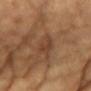follow-up: no biopsy performed (imaged during a skin exam)
subject: female, about 70 years old
illumination: cross-polarized illumination
location: the chest
TBP lesion metrics: a lesion area of about 6.5 mm², an outline eccentricity of about 0.9 (0 = round, 1 = elongated), and a shape-asymmetry score of about 0.25 (0 = symmetric)
size: about 4 mm
image: ~15 mm crop, total-body skin-cancer survey